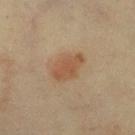The lesion was photographed on a routine skin check and not biopsied; there is no pathology result.
Captured under cross-polarized illumination.
Longest diameter approximately 4 mm.
A female subject, aged 63–67.
A region of skin cropped from a whole-body photographic capture, roughly 15 mm wide.
From the right lower leg.